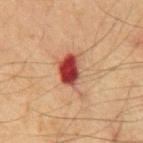Imaged during a routine full-body skin examination; the lesion was not biopsied and no histopathology is available. Approximately 5.5 mm at its widest. The patient is a male aged around 65. A 15 mm close-up tile from a total-body photography series done for melanoma screening. An algorithmic analysis of the crop reported a lesion area of about 11 mm², a shape eccentricity near 0.8, and a symmetry-axis asymmetry near 0.35. It also reported a classifier nevus-likeness of about 0/100 and lesion-presence confidence of about 100/100. Imaged with cross-polarized lighting. The lesion is located on the chest.